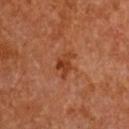Imaged during a routine full-body skin examination; the lesion was not biopsied and no histopathology is available. A lesion tile, about 15 mm wide, cut from a 3D total-body photograph. Located on the chest. The recorded lesion diameter is about 3.5 mm. The patient is a female aged 63–67.